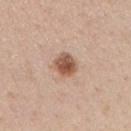Approximately 3 mm at its widest. The lesion is located on the chest. A male subject aged around 40. Cropped from a total-body skin-imaging series; the visible field is about 15 mm. This is a white-light tile. The total-body-photography lesion software estimated a footprint of about 6.5 mm². It also reported a border-irregularity index near 1.5/10, internal color variation of about 4.5 on a 0–10 scale, and radial color variation of about 1.5.